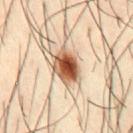No biopsy was performed on this lesion — it was imaged during a full skin examination and was not determined to be concerning. A male patient, aged 38–42. On the front of the torso. A 15 mm crop from a total-body photograph taken for skin-cancer surveillance. This is a cross-polarized tile. The lesion's longest dimension is about 4.5 mm. An algorithmic analysis of the crop reported an average lesion color of about L≈56 a*≈21 b*≈34 (CIELAB), about 22 CIELAB-L* units darker than the surrounding skin, and a normalized border contrast of about 13.5. The analysis additionally found an automated nevus-likeness rating near 100 out of 100 and lesion-presence confidence of about 100/100.